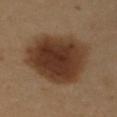{
  "lighting": "cross-polarized",
  "image": {
    "source": "total-body photography crop",
    "field_of_view_mm": 15
  },
  "patient": {
    "sex": "female",
    "age_approx": 50
  },
  "automated_metrics": {
    "area_mm2_approx": 43.0,
    "shape_asymmetry": 0.15,
    "vs_skin_darker_L": 14.0,
    "nevus_likeness_0_100": 100
  },
  "lesion_size": {
    "long_diameter_mm_approx": 8.0
  },
  "site": "arm"
}